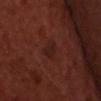| feature | finding |
|---|---|
| follow-up | imaged on a skin check; not biopsied |
| lesion size | ≈2.5 mm |
| automated metrics | an average lesion color of about L≈20 a*≈20 b*≈21 (CIELAB), a lesion–skin lightness drop of about 5, and a normalized lesion–skin contrast near 6; a border-irregularity rating of about 3/10 and a within-lesion color-variation index near 1.5/10 |
| site | the chest |
| lighting | cross-polarized |
| patient | male, in their 50s |
| imaging modality | ~15 mm crop, total-body skin-cancer survey |